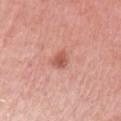  biopsy_status: not biopsied; imaged during a skin examination
  image:
    source: total-body photography crop
    field_of_view_mm: 15
  automated_metrics:
    area_mm2_approx: 3.5
    shape_asymmetry: 0.25
    nevus_likeness_0_100: 50
  lighting: white-light
  patient:
    sex: female
    age_approx: 55
  site: left upper arm